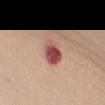{"biopsy_status": "not biopsied; imaged during a skin examination", "site": "front of the torso", "patient": {"sex": "female", "age_approx": 40}, "lesion_size": {"long_diameter_mm_approx": 4.0}, "image": {"source": "total-body photography crop", "field_of_view_mm": 15}, "automated_metrics": {"area_mm2_approx": 7.0, "shape_asymmetry": 0.2, "vs_skin_contrast_norm": 11.5}, "lighting": "white-light"}